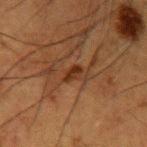| key | value |
|---|---|
| acquisition | ~15 mm crop, total-body skin-cancer survey |
| subject | male, roughly 50 years of age |
| anatomic site | the left upper arm |